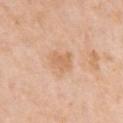No biopsy was performed on this lesion — it was imaged during a full skin examination and was not determined to be concerning.
Approximately 3 mm at its widest.
The lesion is on the left upper arm.
A close-up tile cropped from a whole-body skin photograph, about 15 mm across.
Automated tile analysis of the lesion measured a footprint of about 5 mm² and two-axis asymmetry of about 0.35. The software also gave a border-irregularity index near 3.5/10, a within-lesion color-variation index near 1.5/10, and a peripheral color-asymmetry measure near 0.5.
The patient is a female approximately 60 years of age.
This is a white-light tile.